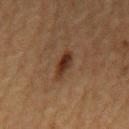Case summary:
* biopsy status — imaged on a skin check; not biopsied
* subject — male, in their mid- to late 80s or older
* image — ~15 mm crop, total-body skin-cancer survey
* body site — the chest
* lesion size — ~3.5 mm (longest diameter)